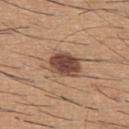notes: no biopsy performed (imaged during a skin exam)
subject: male, about 60 years old
body site: the upper back
illumination: white-light
diameter: about 4 mm
imaging modality: total-body-photography crop, ~15 mm field of view